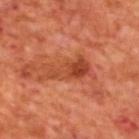Captured during whole-body skin photography for melanoma surveillance; the lesion was not biopsied. From the upper back. Captured under cross-polarized illumination. Cropped from a whole-body photographic skin survey; the tile spans about 15 mm. The patient is a male aged 68–72. Longest diameter approximately 5 mm.